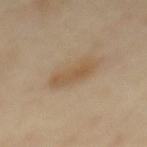Case summary:
* workup: catalogued during a skin exam; not biopsied
* body site: the upper back
* image: ~15 mm crop, total-body skin-cancer survey
* subject: female, aged approximately 45